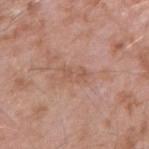Image and clinical context: A male subject aged 73 to 77. Cropped from a total-body skin-imaging series; the visible field is about 15 mm. About 3 mm across. Imaged with white-light lighting. The lesion-visualizer software estimated a lesion area of about 3.5 mm². The analysis additionally found a lesion color around L≈55 a*≈21 b*≈29 in CIELAB, a lesion–skin lightness drop of about 7, and a normalized lesion–skin contrast near 5. It also reported a border-irregularity index near 5/10, a within-lesion color-variation index near 0/10, and a peripheral color-asymmetry measure near 0. And it measured an automated nevus-likeness rating near 0 out of 100 and lesion-presence confidence of about 100/100. The lesion is located on the right forearm.